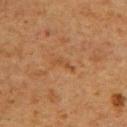Impression: The lesion was photographed on a routine skin check and not biopsied; there is no pathology result. Context: The lesion is located on the upper back. A female subject in their 60s. A roughly 15 mm field-of-view crop from a total-body skin photograph.